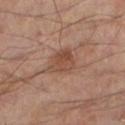Part of a total-body skin-imaging series; this lesion was reviewed on a skin check and was not flagged for biopsy. Automated tile analysis of the lesion measured an average lesion color of about L≈44 a*≈19 b*≈26 (CIELAB), a lesion–skin lightness drop of about 8, and a lesion-to-skin contrast of about 6.5 (normalized; higher = more distinct). This is a cross-polarized tile. A 15 mm close-up extracted from a 3D total-body photography capture. Located on the left lower leg. Longest diameter approximately 3.5 mm. A male patient, approximately 55 years of age.